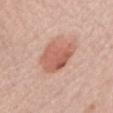Part of a total-body skin-imaging series; this lesion was reviewed on a skin check and was not flagged for biopsy.
The subject is a female aged around 50.
The tile uses white-light illumination.
A 15 mm crop from a total-body photograph taken for skin-cancer surveillance.
The lesion is located on the front of the torso.
The lesion's longest dimension is about 5 mm.